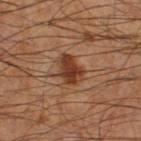The patient is a male approximately 60 years of age.
The lesion is located on the right thigh.
This image is a 15 mm lesion crop taken from a total-body photograph.
About 3.5 mm across.
The lesion-visualizer software estimated a lesion color around L≈33 a*≈21 b*≈28 in CIELAB and a lesion–skin lightness drop of about 12. The software also gave border irregularity of about 3 on a 0–10 scale, a color-variation rating of about 2/10, and radial color variation of about 0.5. The analysis additionally found an automated nevus-likeness rating near 90 out of 100 and a detector confidence of about 100 out of 100 that the crop contains a lesion.